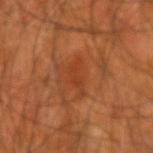biopsy_status: not biopsied; imaged during a skin examination
lesion_size:
  long_diameter_mm_approx: 3.5
site: right forearm
automated_metrics:
  cielab_L: 37
  cielab_a: 27
  cielab_b: 36
  vs_skin_darker_L: 6.0
  vs_skin_contrast_norm: 5.0
  color_variation_0_10: 1.5
patient:
  sex: male
  age_approx: 55
image:
  source: total-body photography crop
  field_of_view_mm: 15
lighting: cross-polarized